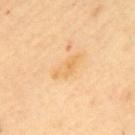Captured during whole-body skin photography for melanoma surveillance; the lesion was not biopsied.
This image is a 15 mm lesion crop taken from a total-body photograph.
The subject is a female aged around 60.
Located on the upper back.